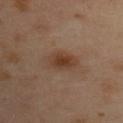• workup: catalogued during a skin exam; not biopsied
• image: ~15 mm crop, total-body skin-cancer survey
• subject: female, aged 38 to 42
• site: the back
• illumination: cross-polarized illumination
• TBP lesion metrics: an outline eccentricity of about 0.8 (0 = round, 1 = elongated); a mean CIELAB color near L≈40 a*≈18 b*≈29, roughly 9 lightness units darker than nearby skin, and a lesion-to-skin contrast of about 8 (normalized; higher = more distinct); a classifier nevus-likeness of about 85/100 and a lesion-detection confidence of about 100/100